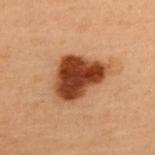{"image": {"source": "total-body photography crop", "field_of_view_mm": 15}, "lesion_size": {"long_diameter_mm_approx": 6.0}, "site": "upper back", "patient": {"sex": "female", "age_approx": 50}, "lighting": "cross-polarized"}